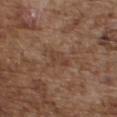workup: catalogued during a skin exam; not biopsied | location: the chest | acquisition: ~15 mm crop, total-body skin-cancer survey | diameter: ~2.5 mm (longest diameter) | image-analysis metrics: an area of roughly 3 mm², a shape eccentricity near 0.85, and a symmetry-axis asymmetry near 0.4; a normalized lesion–skin contrast near 5.5; border irregularity of about 4.5 on a 0–10 scale, a within-lesion color-variation index near 0/10, and radial color variation of about 0; a nevus-likeness score of about 0/100 and a detector confidence of about 100 out of 100 that the crop contains a lesion | tile lighting: white-light illumination | patient: male, approximately 75 years of age.